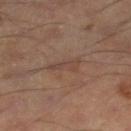| feature | finding |
|---|---|
| follow-up | no biopsy performed (imaged during a skin exam) |
| acquisition | 15 mm crop, total-body photography |
| anatomic site | the right lower leg |
| patient | male, in their mid-50s |
| tile lighting | cross-polarized illumination |